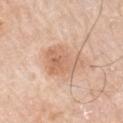Case summary:
• notes: catalogued during a skin exam; not biopsied
• image source: 15 mm crop, total-body photography
• lesion diameter: ≈5 mm
• subject: male, roughly 80 years of age
• automated lesion analysis: a mean CIELAB color near L≈65 a*≈21 b*≈33, about 9 CIELAB-L* units darker than the surrounding skin, and a normalized border contrast of about 6.5; border irregularity of about 3.5 on a 0–10 scale, internal color variation of about 4 on a 0–10 scale, and radial color variation of about 1.5; a detector confidence of about 100 out of 100 that the crop contains a lesion
• anatomic site: the arm